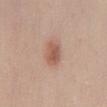{
  "biopsy_status": "not biopsied; imaged during a skin examination",
  "image": {
    "source": "total-body photography crop",
    "field_of_view_mm": 15
  },
  "lesion_size": {
    "long_diameter_mm_approx": 3.0
  },
  "patient": {
    "sex": "male",
    "age_approx": 30
  },
  "site": "abdomen",
  "lighting": "white-light"
}